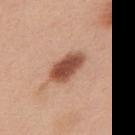Case summary:
* notes · no biopsy performed (imaged during a skin exam)
* location · the front of the torso
* image source · ~15 mm crop, total-body skin-cancer survey
* lighting · white-light illumination
* patient · male, aged around 50
* automated lesion analysis · a shape eccentricity near 0.85 and two-axis asymmetry of about 0.15
* diameter · ~4.5 mm (longest diameter)